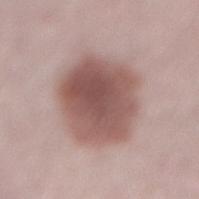follow-up — no biopsy performed (imaged during a skin exam)
anatomic site — the lower back
patient — male, aged 53 to 57
automated lesion analysis — an outline eccentricity of about 0.6 (0 = round, 1 = elongated) and a shape-asymmetry score of about 0.15 (0 = symmetric); a mean CIELAB color near L≈53 a*≈20 b*≈22, about 14 CIELAB-L* units darker than the surrounding skin, and a normalized lesion–skin contrast near 9.5
image — ~15 mm tile from a whole-body skin photo
lighting — white-light illumination